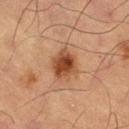Clinical impression:
No biopsy was performed on this lesion — it was imaged during a full skin examination and was not determined to be concerning.